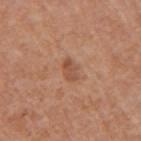• notes · no biopsy performed (imaged during a skin exam)
• body site · the arm
• image · ~15 mm tile from a whole-body skin photo
• illumination · white-light illumination
• subject · female, approximately 55 years of age
• lesion size · about 2.5 mm